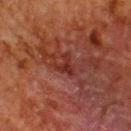A male subject aged around 60. A region of skin cropped from a whole-body photographic capture, roughly 15 mm wide. The tile uses cross-polarized illumination. An algorithmic analysis of the crop reported a footprint of about 4 mm², an outline eccentricity of about 0.45 (0 = round, 1 = elongated), and a symmetry-axis asymmetry near 0.6. The software also gave a border-irregularity rating of about 6/10, a within-lesion color-variation index near 1/10, and a peripheral color-asymmetry measure near 0.5. The analysis additionally found a classifier nevus-likeness of about 0/100. The lesion is located on the back. Measured at roughly 2.5 mm in maximum diameter.